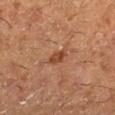The lesion was tiled from a total-body skin photograph and was not biopsied.
A 15 mm crop from a total-body photograph taken for skin-cancer surveillance.
On the right lower leg.
The patient is a female roughly 50 years of age.
About 2.5 mm across.
This is a cross-polarized tile.